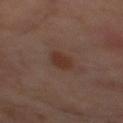Impression: No biopsy was performed on this lesion — it was imaged during a full skin examination and was not determined to be concerning. Background: A male patient, aged around 60. A 15 mm close-up tile from a total-body photography series done for melanoma screening. From the mid back. The lesion's longest dimension is about 3.5 mm. This is a cross-polarized tile.